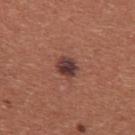Q: Is there a histopathology result?
A: imaged on a skin check; not biopsied
Q: How large is the lesion?
A: about 2.5 mm
Q: Lesion location?
A: the chest
Q: Patient demographics?
A: female, aged 33 to 37
Q: What lighting was used for the tile?
A: white-light illumination
Q: What kind of image is this?
A: 15 mm crop, total-body photography